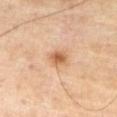- biopsy status · imaged on a skin check; not biopsied
- image source · total-body-photography crop, ~15 mm field of view
- anatomic site · the right thigh
- size · ~2.5 mm (longest diameter)
- patient · male, about 70 years old
- tile lighting · cross-polarized illumination